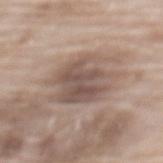{
  "biopsy_status": "not biopsied; imaged during a skin examination",
  "patient": {
    "sex": "female",
    "age_approx": 75
  },
  "lesion_size": {
    "long_diameter_mm_approx": 6.5
  },
  "site": "mid back",
  "lighting": "white-light",
  "image": {
    "source": "total-body photography crop",
    "field_of_view_mm": 15
  }
}